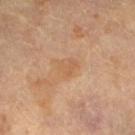site: left lower leg
image:
  source: total-body photography crop
  field_of_view_mm: 15
lighting: cross-polarized
patient:
  sex: female
  age_approx: 70
lesion_size:
  long_diameter_mm_approx: 2.5